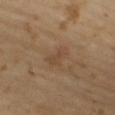Case summary:
• biopsy status — no biopsy performed (imaged during a skin exam)
• image-analysis metrics — a footprint of about 5 mm², a shape eccentricity near 0.9, and two-axis asymmetry of about 0.35; a mean CIELAB color near L≈43 a*≈16 b*≈29, a lesion–skin lightness drop of about 6, and a lesion-to-skin contrast of about 5 (normalized; higher = more distinct); a border-irregularity index near 4/10, a within-lesion color-variation index near 4/10, and peripheral color asymmetry of about 1.5; a nevus-likeness score of about 0/100 and lesion-presence confidence of about 100/100
• image — ~15 mm crop, total-body skin-cancer survey
• patient — male, in their mid- to late 60s
• lesion diameter — about 3.5 mm